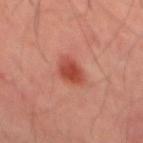The lesion was tiled from a total-body skin photograph and was not biopsied. Captured under cross-polarized illumination. Located on the mid back. A male patient, aged around 45. A 15 mm close-up extracted from a 3D total-body photography capture.